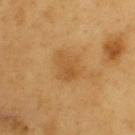Q: Is there a histopathology result?
A: catalogued during a skin exam; not biopsied
Q: What did automated image analysis measure?
A: two-axis asymmetry of about 0.4; an average lesion color of about L≈52 a*≈20 b*≈42 (CIELAB), a lesion–skin lightness drop of about 7, and a normalized lesion–skin contrast near 5
Q: What is the anatomic site?
A: the upper back
Q: What is the lesion's diameter?
A: ~3.5 mm (longest diameter)
Q: Illumination type?
A: cross-polarized
Q: Patient demographics?
A: male, roughly 60 years of age
Q: What kind of image is this?
A: total-body-photography crop, ~15 mm field of view